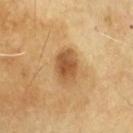Part of a total-body skin-imaging series; this lesion was reviewed on a skin check and was not flagged for biopsy.
Imaged with cross-polarized lighting.
About 4 mm across.
A male patient approximately 65 years of age.
A 15 mm close-up tile from a total-body photography series done for melanoma screening.
From the chest.
The lesion-visualizer software estimated an area of roughly 9 mm² and two-axis asymmetry of about 0.2. The software also gave a lesion color around L≈54 a*≈20 b*≈40 in CIELAB, about 13 CIELAB-L* units darker than the surrounding skin, and a normalized border contrast of about 8.5. The software also gave internal color variation of about 4 on a 0–10 scale and a peripheral color-asymmetry measure near 1. The software also gave a classifier nevus-likeness of about 85/100.